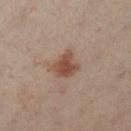body site — the right leg; image — total-body-photography crop, ~15 mm field of view; image-analysis metrics — a border-irregularity index near 3/10, a within-lesion color-variation index near 2.5/10, and peripheral color asymmetry of about 0.5; lighting — cross-polarized illumination; patient — female, aged around 40; diameter — ≈3 mm.